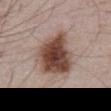| field | value |
|---|---|
| biopsy status | catalogued during a skin exam; not biopsied |
| location | the abdomen |
| lighting | white-light |
| image source | total-body-photography crop, ~15 mm field of view |
| size | ~6.5 mm (longest diameter) |
| patient | male, roughly 55 years of age |